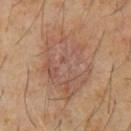{"biopsy_status": "not biopsied; imaged during a skin examination", "automated_metrics": {"cielab_L": 49, "cielab_a": 18, "cielab_b": 26, "vs_skin_contrast_norm": 5.5, "border_irregularity_0_10": 3.5, "color_variation_0_10": 4.5, "nevus_likeness_0_100": 5}, "lesion_size": {"long_diameter_mm_approx": 8.5}, "lighting": "cross-polarized", "patient": {"sex": "male", "age_approx": 60}, "site": "front of the torso", "image": {"source": "total-body photography crop", "field_of_view_mm": 15}}